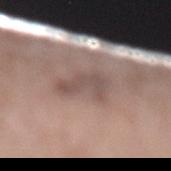<case>
  <biopsy_status>not biopsied; imaged during a skin examination</biopsy_status>
  <automated_metrics>
    <eccentricity>0.8</eccentricity>
    <shape_asymmetry>0.4</shape_asymmetry>
    <border_irregularity_0_10>5.0</border_irregularity_0_10>
    <color_variation_0_10>2.5</color_variation_0_10>
    <peripheral_color_asymmetry>0.5</peripheral_color_asymmetry>
  </automated_metrics>
  <lesion_size>
    <long_diameter_mm_approx>4.0</long_diameter_mm_approx>
  </lesion_size>
  <lighting>white-light</lighting>
  <site>left lower leg</site>
  <image>
    <source>total-body photography crop</source>
    <field_of_view_mm>15</field_of_view_mm>
  </image>
  <patient>
    <sex>female</sex>
    <age_approx>70</age_approx>
  </patient>
</case>